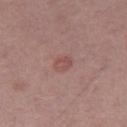The lesion was tiled from a total-body skin photograph and was not biopsied.
This is a white-light tile.
A close-up tile cropped from a whole-body skin photograph, about 15 mm across.
The lesion is located on the right thigh.
A male subject approximately 70 years of age.
The recorded lesion diameter is about 2.5 mm.
An algorithmic analysis of the crop reported a shape eccentricity near 0.65. And it measured a border-irregularity index near 2/10 and radial color variation of about 1.